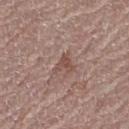On the left thigh. A close-up tile cropped from a whole-body skin photograph, about 15 mm across. A female patient in their mid- to late 60s.